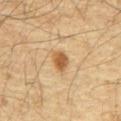The lesion was tiled from a total-body skin photograph and was not biopsied.
A 15 mm close-up extracted from a 3D total-body photography capture.
This is a cross-polarized tile.
A male patient in their mid-60s.
Longest diameter approximately 3 mm.
The lesion is located on the abdomen.